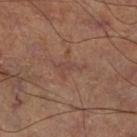Case summary:
- biopsy status: no biopsy performed (imaged during a skin exam)
- anatomic site: the right lower leg
- lighting: cross-polarized
- image source: 15 mm crop, total-body photography